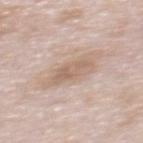{
  "biopsy_status": "not biopsied; imaged during a skin examination",
  "image": {
    "source": "total-body photography crop",
    "field_of_view_mm": 15
  },
  "lighting": "white-light",
  "patient": {
    "sex": "female",
    "age_approx": 65
  },
  "automated_metrics": {
    "area_mm2_approx": 6.0,
    "shape_asymmetry": 0.3,
    "cielab_L": 62,
    "cielab_a": 16,
    "cielab_b": 27,
    "vs_skin_darker_L": 8.0,
    "vs_skin_contrast_norm": 5.5,
    "border_irregularity_0_10": 3.5,
    "color_variation_0_10": 2.5,
    "peripheral_color_asymmetry": 1.0
  },
  "lesion_size": {
    "long_diameter_mm_approx": 4.0
  },
  "site": "upper back"
}